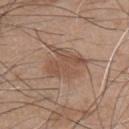{
  "biopsy_status": "not biopsied; imaged during a skin examination"
}